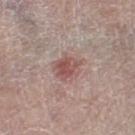Clinical impression: Part of a total-body skin-imaging series; this lesion was reviewed on a skin check and was not flagged for biopsy. Context: A roughly 15 mm field-of-view crop from a total-body skin photograph. On the right thigh. A male subject, aged approximately 80. Measured at roughly 3.5 mm in maximum diameter. This is a white-light tile.